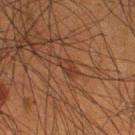Findings:
* workup — total-body-photography surveillance lesion; no biopsy
* image source — ~15 mm tile from a whole-body skin photo
* site — the right thigh
* patient — male, roughly 50 years of age